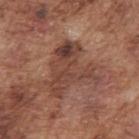Part of a total-body skin-imaging series; this lesion was reviewed on a skin check and was not flagged for biopsy. The lesion-visualizer software estimated an eccentricity of roughly 0.5 and a symmetry-axis asymmetry near 0.55. And it measured a lesion–skin lightness drop of about 9 and a lesion-to-skin contrast of about 7 (normalized; higher = more distinct). The analysis additionally found a border-irregularity rating of about 8/10 and a peripheral color-asymmetry measure near 2.5. Longest diameter approximately 6 mm. A region of skin cropped from a whole-body photographic capture, roughly 15 mm wide. The patient is a male about 75 years old. On the arm.